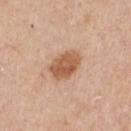The lesion is located on the chest.
Captured under white-light illumination.
A region of skin cropped from a whole-body photographic capture, roughly 15 mm wide.
Automated tile analysis of the lesion measured a lesion area of about 8.5 mm² and a shape eccentricity near 0.75.
The subject is a male approximately 60 years of age.
Longest diameter approximately 4 mm.